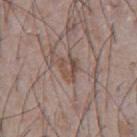{"biopsy_status": "not biopsied; imaged during a skin examination", "lesion_size": {"long_diameter_mm_approx": 3.0}, "lighting": "white-light", "patient": {"sex": "male", "age_approx": 75}, "image": {"source": "total-body photography crop", "field_of_view_mm": 15}, "site": "chest"}